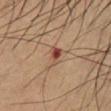notes = no biopsy performed (imaged during a skin exam)
TBP lesion metrics = a mean CIELAB color near L≈45 a*≈22 b*≈28, roughly 11 lightness units darker than nearby skin, and a normalized lesion–skin contrast near 8.5; a border-irregularity index near 3.5/10 and internal color variation of about 4.5 on a 0–10 scale; a nevus-likeness score of about 0/100 and a detector confidence of about 100 out of 100 that the crop contains a lesion
patient = male, aged around 50
lighting = cross-polarized
image = 15 mm crop, total-body photography
anatomic site = the chest
diameter = ~2.5 mm (longest diameter)